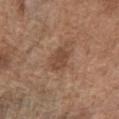Captured during whole-body skin photography for melanoma surveillance; the lesion was not biopsied. A region of skin cropped from a whole-body photographic capture, roughly 15 mm wide. The lesion is located on the chest. The subject is a male in their mid- to late 70s.